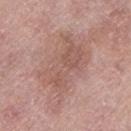No biopsy was performed on this lesion — it was imaged during a full skin examination and was not determined to be concerning. On the left lower leg. This is a white-light tile. Measured at roughly 9 mm in maximum diameter. A close-up tile cropped from a whole-body skin photograph, about 15 mm across. Automated image analysis of the tile measured an average lesion color of about L≈56 a*≈20 b*≈25 (CIELAB), roughly 8 lightness units darker than nearby skin, and a normalized lesion–skin contrast near 5.5. The analysis additionally found a border-irregularity rating of about 5.5/10. The patient is a female roughly 60 years of age.